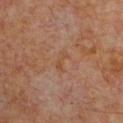This lesion was catalogued during total-body skin photography and was not selected for biopsy.
A male patient, in their 60s.
A region of skin cropped from a whole-body photographic capture, roughly 15 mm wide.
This is a cross-polarized tile.
Located on the upper back.